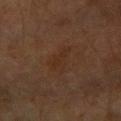– body site: the right forearm
– lesion size: ~3.5 mm (longest diameter)
– subject: female, approximately 70 years of age
– imaging modality: ~15 mm crop, total-body skin-cancer survey
– lighting: cross-polarized